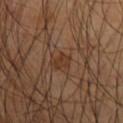Captured during whole-body skin photography for melanoma surveillance; the lesion was not biopsied. A close-up tile cropped from a whole-body skin photograph, about 15 mm across. This is a cross-polarized tile. The recorded lesion diameter is about 2.5 mm. The subject is a male aged around 55. The lesion is located on the right upper arm. Automated image analysis of the tile measured a lesion area of about 3.5 mm² and a shape-asymmetry score of about 0.25 (0 = symmetric). It also reported about 6 CIELAB-L* units darker than the surrounding skin and a normalized lesion–skin contrast near 6.5. It also reported a border-irregularity rating of about 2.5/10 and a color-variation rating of about 2/10. The analysis additionally found an automated nevus-likeness rating near 0 out of 100.